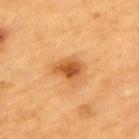Part of a total-body skin-imaging series; this lesion was reviewed on a skin check and was not flagged for biopsy.
A lesion tile, about 15 mm wide, cut from a 3D total-body photograph.
The subject is a male approximately 85 years of age or older.
The lesion is located on the upper back.
The tile uses cross-polarized illumination.